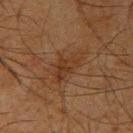Context:
Automated image analysis of the tile measured an average lesion color of about L≈29 a*≈17 b*≈27 (CIELAB), a lesion–skin lightness drop of about 5, and a normalized border contrast of about 6. It also reported a border-irregularity index near 4.5/10, a color-variation rating of about 3/10, and peripheral color asymmetry of about 1. A lesion tile, about 15 mm wide, cut from a 3D total-body photograph. The lesion is located on the right upper arm. Measured at roughly 5 mm in maximum diameter. A male subject, about 65 years old.